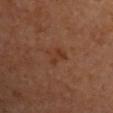Assessment: Imaged during a routine full-body skin examination; the lesion was not biopsied and no histopathology is available. Acquisition and patient details: A 15 mm crop from a total-body photograph taken for skin-cancer surveillance. A male subject about 65 years old. An algorithmic analysis of the crop reported a lesion area of about 4 mm², an outline eccentricity of about 0.7 (0 = round, 1 = elongated), and a shape-asymmetry score of about 0.45 (0 = symmetric). The software also gave border irregularity of about 4.5 on a 0–10 scale, a color-variation rating of about 1.5/10, and peripheral color asymmetry of about 0. This is a cross-polarized tile. The lesion is on the right upper arm.